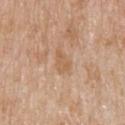Part of a total-body skin-imaging series; this lesion was reviewed on a skin check and was not flagged for biopsy. A lesion tile, about 15 mm wide, cut from a 3D total-body photograph. From the upper back. The lesion's longest dimension is about 3 mm. Imaged with white-light lighting. The patient is a male in their 80s. An algorithmic analysis of the crop reported a shape eccentricity near 0.85 and a shape-asymmetry score of about 0.4 (0 = symmetric). The analysis additionally found a border-irregularity index near 4/10 and radial color variation of about 0.5.